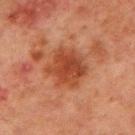<record>
  <biopsy_status>not biopsied; imaged during a skin examination</biopsy_status>
  <lighting>cross-polarized</lighting>
  <automated_metrics>
    <area_mm2_approx>15.0</area_mm2_approx>
    <eccentricity>0.4</eccentricity>
    <cielab_L>37</cielab_L>
    <cielab_a>25</cielab_a>
    <cielab_b>30</cielab_b>
    <vs_skin_darker_L>9.0</vs_skin_darker_L>
    <vs_skin_contrast_norm>8.0</vs_skin_contrast_norm>
    <color_variation_0_10>3.5</color_variation_0_10>
    <peripheral_color_asymmetry>1.0</peripheral_color_asymmetry>
    <nevus_likeness_0_100>65</nevus_likeness_0_100>
  </automated_metrics>
  <lesion_size>
    <long_diameter_mm_approx>4.5</long_diameter_mm_approx>
  </lesion_size>
  <site>chest</site>
  <patient>
    <sex>male</sex>
    <age_approx>65</age_approx>
  </patient>
  <image>
    <source>total-body photography crop</source>
    <field_of_view_mm>15</field_of_view_mm>
  </image>
</record>